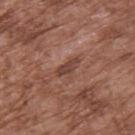Q: Who is the patient?
A: male, aged around 75
Q: What lighting was used for the tile?
A: white-light illumination
Q: What kind of image is this?
A: ~15 mm crop, total-body skin-cancer survey
Q: How large is the lesion?
A: about 3 mm
Q: What is the anatomic site?
A: the back